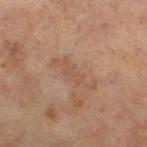workup: no biopsy performed (imaged during a skin exam); location: the right thigh; subject: female, in their 60s; acquisition: ~15 mm tile from a whole-body skin photo.